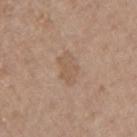This lesion was catalogued during total-body skin photography and was not selected for biopsy.
The patient is a female aged 63 to 67.
The total-body-photography lesion software estimated an outline eccentricity of about 0.85 (0 = round, 1 = elongated) and a shape-asymmetry score of about 0.25 (0 = symmetric). And it measured an average lesion color of about L≈56 a*≈16 b*≈29 (CIELAB), about 6 CIELAB-L* units darker than the surrounding skin, and a normalized lesion–skin contrast near 5. The software also gave a detector confidence of about 100 out of 100 that the crop contains a lesion.
The lesion is on the right upper arm.
Approximately 3.5 mm at its widest.
This image is a 15 mm lesion crop taken from a total-body photograph.